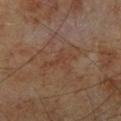<case>
<biopsy_status>not biopsied; imaged during a skin examination</biopsy_status>
<image>
  <source>total-body photography crop</source>
  <field_of_view_mm>15</field_of_view_mm>
</image>
<patient>
  <sex>male</sex>
  <age_approx>70</age_approx>
</patient>
<site>leg</site>
<automated_metrics>
  <color_variation_0_10>1.0</color_variation_0_10>
  <peripheral_color_asymmetry>0.5</peripheral_color_asymmetry>
  <nevus_likeness_0_100>0</nevus_likeness_0_100>
</automated_metrics>
</case>